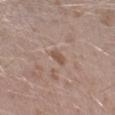Assessment: This lesion was catalogued during total-body skin photography and was not selected for biopsy. Image and clinical context: From the leg. Longest diameter approximately 3 mm. An algorithmic analysis of the crop reported a lesion area of about 4 mm² and a symmetry-axis asymmetry near 0.35. It also reported a classifier nevus-likeness of about 0/100 and a lesion-detection confidence of about 100/100. A male subject roughly 40 years of age. A 15 mm close-up tile from a total-body photography series done for melanoma screening.